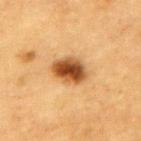illumination: cross-polarized
lesion diameter: ~4.5 mm (longest diameter)
image-analysis metrics: a symmetry-axis asymmetry near 0.1; a classifier nevus-likeness of about 100/100 and a lesion-detection confidence of about 100/100
subject: male, about 85 years old or older
acquisition: total-body-photography crop, ~15 mm field of view
body site: the upper back